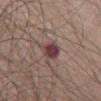Imaged during a routine full-body skin examination; the lesion was not biopsied and no histopathology is available.
The patient is a male approximately 65 years of age.
Measured at roughly 3 mm in maximum diameter.
A 15 mm close-up tile from a total-body photography series done for melanoma screening.
Captured under white-light illumination.
The lesion is on the abdomen.